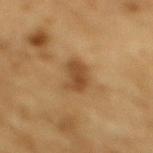<tbp_lesion>
<biopsy_status>not biopsied; imaged during a skin examination</biopsy_status>
<automated_metrics>
  <border_irregularity_0_10>3.0</border_irregularity_0_10>
  <peripheral_color_asymmetry>1.0</peripheral_color_asymmetry>
  <nevus_likeness_0_100>30</nevus_likeness_0_100>
  <lesion_detection_confidence_0_100>100</lesion_detection_confidence_0_100>
</automated_metrics>
<lighting>cross-polarized</lighting>
<site>mid back</site>
<image>
  <source>total-body photography crop</source>
  <field_of_view_mm>15</field_of_view_mm>
</image>
<patient>
  <sex>male</sex>
  <age_approx>85+</age_approx>
</patient>
</tbp_lesion>